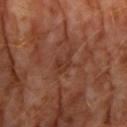The lesion was tiled from a total-body skin photograph and was not biopsied.
The tile uses cross-polarized illumination.
Approximately 3 mm at its widest.
A 15 mm crop from a total-body photograph taken for skin-cancer surveillance.
Located on the arm.
A male patient, aged approximately 60.